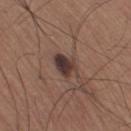Impression:
Imaged during a routine full-body skin examination; the lesion was not biopsied and no histopathology is available.
Acquisition and patient details:
An algorithmic analysis of the crop reported an automated nevus-likeness rating near 90 out of 100 and a detector confidence of about 100 out of 100 that the crop contains a lesion. Captured under white-light illumination. A male subject aged 58–62. The recorded lesion diameter is about 3 mm. A 15 mm close-up extracted from a 3D total-body photography capture. On the right thigh.